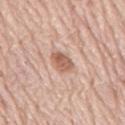<record>
  <biopsy_status>not biopsied; imaged during a skin examination</biopsy_status>
  <patient>
    <sex>male</sex>
    <age_approx>80</age_approx>
  </patient>
  <lesion_size>
    <long_diameter_mm_approx>3.0</long_diameter_mm_approx>
  </lesion_size>
  <lighting>white-light</lighting>
  <image>
    <source>total-body photography crop</source>
    <field_of_view_mm>15</field_of_view_mm>
  </image>
  <site>mid back</site>
  <automated_metrics>
    <area_mm2_approx>5.0</area_mm2_approx>
    <vs_skin_contrast_norm>8.0</vs_skin_contrast_norm>
    <border_irregularity_0_10>2.0</border_irregularity_0_10>
    <color_variation_0_10>3.5</color_variation_0_10>
    <peripheral_color_asymmetry>1.5</peripheral_color_asymmetry>
    <nevus_likeness_0_100>95</nevus_likeness_0_100>
  </automated_metrics>
</record>